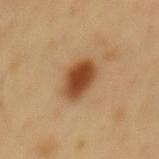| feature | finding |
|---|---|
| notes | total-body-photography surveillance lesion; no biopsy |
| location | the back |
| subject | male, about 50 years old |
| lesion diameter | about 4.5 mm |
| acquisition | ~15 mm tile from a whole-body skin photo |